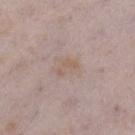{"biopsy_status": "not biopsied; imaged during a skin examination", "image": {"source": "total-body photography crop", "field_of_view_mm": 15}, "automated_metrics": {"eccentricity": 0.8, "cielab_L": 58, "cielab_a": 15, "cielab_b": 25, "vs_skin_darker_L": 6.0, "vs_skin_contrast_norm": 5.0}, "patient": {"sex": "female", "age_approx": 30}, "site": "right lower leg"}